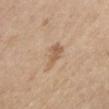  biopsy_status: not biopsied; imaged during a skin examination
  patient:
    sex: female
    age_approx: 65
  site: chest
  image:
    source: total-body photography crop
    field_of_view_mm: 15